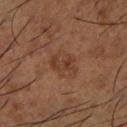Part of a total-body skin-imaging series; this lesion was reviewed on a skin check and was not flagged for biopsy. The recorded lesion diameter is about 3.5 mm. Located on the left lower leg. A 15 mm close-up tile from a total-body photography series done for melanoma screening. A male patient, about 65 years old. This is a cross-polarized tile.